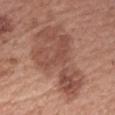follow-up — catalogued during a skin exam; not biopsied
lesion size — about 10 mm
patient — female, approximately 60 years of age
image source — 15 mm crop, total-body photography
body site — the right forearm